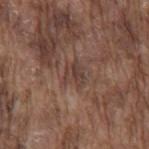– workup: imaged on a skin check; not biopsied
– patient: male, roughly 75 years of age
– automated lesion analysis: an area of roughly 5 mm², an outline eccentricity of about 0.45 (0 = round, 1 = elongated), and a shape-asymmetry score of about 0.35 (0 = symmetric); a lesion color around L≈40 a*≈17 b*≈22 in CIELAB, a lesion–skin lightness drop of about 7, and a normalized border contrast of about 6; a border-irregularity rating of about 4/10, internal color variation of about 4 on a 0–10 scale, and radial color variation of about 1.5; lesion-presence confidence of about 65/100
– image source: total-body-photography crop, ~15 mm field of view
– body site: the mid back
– lighting: white-light illumination